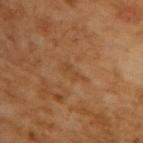{"lighting": "cross-polarized", "image": {"source": "total-body photography crop", "field_of_view_mm": 15}, "patient": {"sex": "male", "age_approx": 65}, "automated_metrics": {"cielab_L": 43, "cielab_a": 21, "cielab_b": 36, "vs_skin_darker_L": 5.0, "vs_skin_contrast_norm": 4.5, "border_irregularity_0_10": 6.0, "color_variation_0_10": 0.0, "peripheral_color_asymmetry": 0.0, "nevus_likeness_0_100": 0, "lesion_detection_confidence_0_100": 100}, "lesion_size": {"long_diameter_mm_approx": 3.0}}